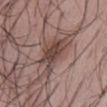Captured during whole-body skin photography for melanoma surveillance; the lesion was not biopsied.